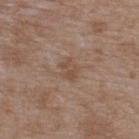Part of a total-body skin-imaging series; this lesion was reviewed on a skin check and was not flagged for biopsy. Located on the back. A male patient aged around 50. The tile uses white-light illumination. About 2.5 mm across. Cropped from a total-body skin-imaging series; the visible field is about 15 mm.